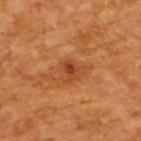Clinical impression: No biopsy was performed on this lesion — it was imaged during a full skin examination and was not determined to be concerning. Image and clinical context: A male patient, approximately 65 years of age. The recorded lesion diameter is about 3 mm. A lesion tile, about 15 mm wide, cut from a 3D total-body photograph.